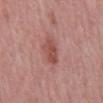Impression: The lesion was tiled from a total-body skin photograph and was not biopsied. Context: The lesion is on the mid back. This image is a 15 mm lesion crop taken from a total-body photograph. Approximately 4 mm at its widest. A male patient aged approximately 50.